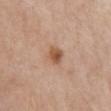workup = no biopsy performed (imaged during a skin exam) | patient = female, about 60 years old | image source = ~15 mm tile from a whole-body skin photo | image-analysis metrics = a mean CIELAB color near L≈54 a*≈21 b*≈32 and a lesion–skin lightness drop of about 11 | body site = the chest.